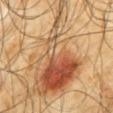| feature | finding |
|---|---|
| anatomic site | the back |
| image | ~15 mm tile from a whole-body skin photo |
| patient | male, approximately 65 years of age |
| automated metrics | an average lesion color of about L≈52 a*≈21 b*≈35 (CIELAB), a lesion–skin lightness drop of about 13, and a normalized lesion–skin contrast near 9; a within-lesion color-variation index near 10/10 and radial color variation of about 3 |
| lesion diameter | about 14.5 mm |